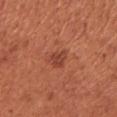Q: Was a biopsy performed?
A: no biopsy performed (imaged during a skin exam)
Q: Lesion location?
A: the right upper arm
Q: What kind of image is this?
A: total-body-photography crop, ~15 mm field of view
Q: What did automated image analysis measure?
A: a footprint of about 4 mm², a shape eccentricity near 0.65, and a symmetry-axis asymmetry near 0.4; a lesion color around L≈44 a*≈29 b*≈32 in CIELAB and a lesion-to-skin contrast of about 6.5 (normalized; higher = more distinct); a border-irregularity rating of about 3.5/10, a color-variation rating of about 1.5/10, and radial color variation of about 0.5; a nevus-likeness score of about 40/100 and lesion-presence confidence of about 100/100
Q: Patient demographics?
A: female, aged approximately 65
Q: Illumination type?
A: white-light illumination